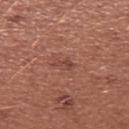Q: Was this lesion biopsied?
A: imaged on a skin check; not biopsied
Q: Patient demographics?
A: female, roughly 35 years of age
Q: Illumination type?
A: white-light
Q: How large is the lesion?
A: ~2.5 mm (longest diameter)
Q: What kind of image is this?
A: ~15 mm tile from a whole-body skin photo
Q: Where on the body is the lesion?
A: the right forearm